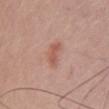Recorded during total-body skin imaging; not selected for excision or biopsy.